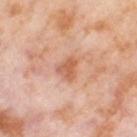  site: right thigh
  image:
    source: total-body photography crop
    field_of_view_mm: 15
  patient:
    sex: female
    age_approx: 55